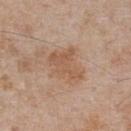The lesion was tiled from a total-body skin photograph and was not biopsied.
The tile uses white-light illumination.
From the chest.
The subject is a male aged 63 to 67.
A 15 mm close-up tile from a total-body photography series done for melanoma screening.
Measured at roughly 4.5 mm in maximum diameter.